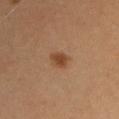Case summary:
– follow-up — imaged on a skin check; not biopsied
– diameter — about 2.5 mm
– body site — the left upper arm
– automated lesion analysis — a lesion area of about 4 mm², an outline eccentricity of about 0.6 (0 = round, 1 = elongated), and two-axis asymmetry of about 0.2
– acquisition — ~15 mm tile from a whole-body skin photo
– subject — male, aged 28–32